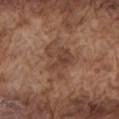About 4.5 mm across. On the left upper arm. Imaged with white-light lighting. A lesion tile, about 15 mm wide, cut from a 3D total-body photograph. The subject is a male roughly 75 years of age.